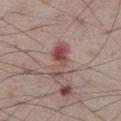Captured during whole-body skin photography for melanoma surveillance; the lesion was not biopsied. The lesion's longest dimension is about 4.5 mm. Captured under white-light illumination. A 15 mm close-up tile from a total-body photography series done for melanoma screening. The lesion is located on the right thigh. A male subject aged around 75.